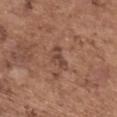| key | value |
|---|---|
| biopsy status | no biopsy performed (imaged during a skin exam) |
| body site | the mid back |
| size | ~3 mm (longest diameter) |
| subject | female, aged approximately 65 |
| image source | 15 mm crop, total-body photography |
| lighting | white-light |